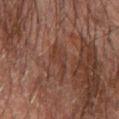* follow-up — catalogued during a skin exam; not biopsied
* tile lighting — cross-polarized illumination
* subject — male, in their 70s
* body site — the right forearm
* TBP lesion metrics — a shape eccentricity near 0.8 and a symmetry-axis asymmetry near 0.6; a border-irregularity index near 6.5/10, a within-lesion color-variation index near 3/10, and a peripheral color-asymmetry measure near 1; a classifier nevus-likeness of about 0/100
* image source — ~15 mm tile from a whole-body skin photo